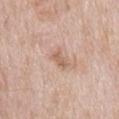Q: Is there a histopathology result?
A: total-body-photography surveillance lesion; no biopsy
Q: What are the patient's age and sex?
A: male, in their mid-60s
Q: Lesion location?
A: the mid back
Q: What is the imaging modality?
A: ~15 mm crop, total-body skin-cancer survey
Q: How was the tile lit?
A: white-light